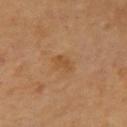Clinical impression:
Recorded during total-body skin imaging; not selected for excision or biopsy.
Acquisition and patient details:
A 15 mm crop from a total-body photograph taken for skin-cancer surveillance. The subject is aged around 60. On the right upper arm. The total-body-photography lesion software estimated a within-lesion color-variation index near 1/10 and radial color variation of about 0.5. Imaged with cross-polarized lighting. The lesion's longest dimension is about 3 mm.